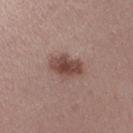Assessment:
Recorded during total-body skin imaging; not selected for excision or biopsy.